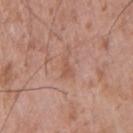Case summary:
- biopsy status — catalogued during a skin exam; not biopsied
- acquisition — ~15 mm crop, total-body skin-cancer survey
- patient — male, in their 50s
- TBP lesion metrics — a lesion–skin lightness drop of about 6 and a normalized border contrast of about 4.5; a border-irregularity index near 5/10, a within-lesion color-variation index near 1/10, and peripheral color asymmetry of about 0.5
- body site — the right upper arm
- lesion diameter — about 3 mm